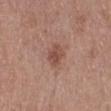Case summary:
- notes: catalogued during a skin exam; not biopsied
- image: ~15 mm tile from a whole-body skin photo
- anatomic site: the right lower leg
- patient: female, approximately 50 years of age